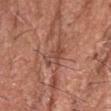The lesion was tiled from a total-body skin photograph and was not biopsied. Located on the head or neck. A male subject, approximately 80 years of age. This is a white-light tile. Longest diameter approximately 3 mm. A lesion tile, about 15 mm wide, cut from a 3D total-body photograph.